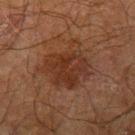The lesion was photographed on a routine skin check and not biopsied; there is no pathology result. Cropped from a total-body skin-imaging series; the visible field is about 15 mm. Longest diameter approximately 6 mm. Imaged with cross-polarized lighting. From the left upper arm. A male patient aged approximately 70. The total-body-photography lesion software estimated an area of roughly 20 mm², an eccentricity of roughly 0.45, and a shape-asymmetry score of about 0.3 (0 = symmetric). It also reported a mean CIELAB color near L≈27 a*≈18 b*≈25, about 7 CIELAB-L* units darker than the surrounding skin, and a normalized lesion–skin contrast near 7.5. It also reported a border-irregularity index near 4/10 and a color-variation rating of about 3.5/10.